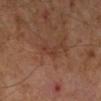Notes:
* workup: total-body-photography surveillance lesion; no biopsy
* image source: 15 mm crop, total-body photography
* body site: the right lower leg
* subject: male, in their 60s